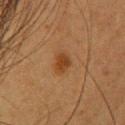{"biopsy_status": "not biopsied; imaged during a skin examination", "site": "head or neck", "lesion_size": {"long_diameter_mm_approx": 2.5}, "image": {"source": "total-body photography crop", "field_of_view_mm": 15}, "lighting": "cross-polarized", "patient": {"sex": "female", "age_approx": 40}}